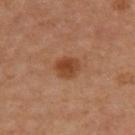Case summary:
– biopsy status · imaged on a skin check; not biopsied
– image source · ~15 mm crop, total-body skin-cancer survey
– patient · female, in their mid-40s
– lighting · cross-polarized illumination
– anatomic site · the back